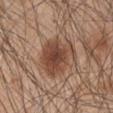Q: Was a biopsy performed?
A: total-body-photography surveillance lesion; no biopsy
Q: What did automated image analysis measure?
A: a border-irregularity index near 3.5/10 and a within-lesion color-variation index near 5/10
Q: How was this image acquired?
A: ~15 mm tile from a whole-body skin photo
Q: Where on the body is the lesion?
A: the right upper arm
Q: What are the patient's age and sex?
A: male, roughly 45 years of age
Q: What lighting was used for the tile?
A: white-light illumination
Q: How large is the lesion?
A: ≈5.5 mm